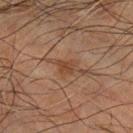Case summary:
* follow-up — no biopsy performed (imaged during a skin exam)
* body site — the arm
* subject — male, in their 70s
* acquisition — ~15 mm tile from a whole-body skin photo